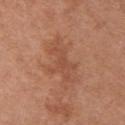follow-up: imaged on a skin check; not biopsied
tile lighting: white-light
patient: female, in their mid- to late 60s
acquisition: total-body-photography crop, ~15 mm field of view
location: the left upper arm
lesion size: ~5.5 mm (longest diameter)